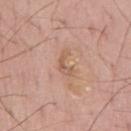biopsy_status: not biopsied; imaged during a skin examination
site: mid back
lesion_size:
  long_diameter_mm_approx: 3.0
image:
  source: total-body photography crop
  field_of_view_mm: 15
lighting: white-light
patient:
  sex: male
  age_approx: 65